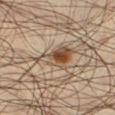The lesion was tiled from a total-body skin photograph and was not biopsied. A 15 mm crop from a total-body photograph taken for skin-cancer surveillance. The lesion is on the left lower leg. Captured under cross-polarized illumination. Automated tile analysis of the lesion measured a lesion color around L≈44 a*≈15 b*≈27 in CIELAB, roughly 11 lightness units darker than nearby skin, and a normalized lesion–skin contrast near 9. The recorded lesion diameter is about 4.5 mm. A male patient aged 33–37.